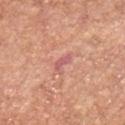Clinical impression:
No biopsy was performed on this lesion — it was imaged during a full skin examination and was not determined to be concerning.
Clinical summary:
The patient is a male aged 63–67. Measured at roughly 2.5 mm in maximum diameter. An algorithmic analysis of the crop reported a symmetry-axis asymmetry near 0.35. The software also gave a mean CIELAB color near L≈59 a*≈29 b*≈24, a lesion–skin lightness drop of about 8, and a normalized lesion–skin contrast near 7. It also reported a border-irregularity rating of about 4/10 and peripheral color asymmetry of about 0. The software also gave a nevus-likeness score of about 0/100 and lesion-presence confidence of about 100/100. A region of skin cropped from a whole-body photographic capture, roughly 15 mm wide. On the chest. Captured under white-light illumination.